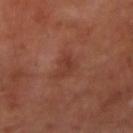follow-up — imaged on a skin check; not biopsied
image — ~15 mm crop, total-body skin-cancer survey
diameter — ≈3.5 mm
tile lighting — cross-polarized illumination
body site — the arm
subject — male, aged approximately 50